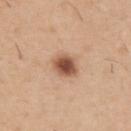location=the back
acquisition=total-body-photography crop, ~15 mm field of view
subject=male, about 40 years old